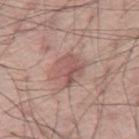Impression:
The lesion was tiled from a total-body skin photograph and was not biopsied.
Context:
A male subject, aged 68–72. A 15 mm close-up extracted from a 3D total-body photography capture. The lesion is on the leg.